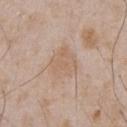The lesion was tiled from a total-body skin photograph and was not biopsied.
On the chest.
Captured under white-light illumination.
The subject is a male approximately 50 years of age.
Approximately 3.5 mm at its widest.
A region of skin cropped from a whole-body photographic capture, roughly 15 mm wide.